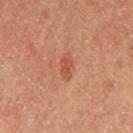workup = imaged on a skin check; not biopsied
acquisition = ~15 mm tile from a whole-body skin photo
subject = female
body site = the left thigh